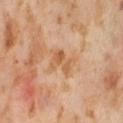Assessment: Captured during whole-body skin photography for melanoma surveillance; the lesion was not biopsied. Acquisition and patient details: A female subject, approximately 55 years of age. This is a cross-polarized tile. The lesion is located on the abdomen. Approximately 3.5 mm at its widest. Cropped from a whole-body photographic skin survey; the tile spans about 15 mm. The total-body-photography lesion software estimated about 8 CIELAB-L* units darker than the surrounding skin. The software also gave border irregularity of about 4 on a 0–10 scale, a color-variation rating of about 4.5/10, and peripheral color asymmetry of about 1.5.